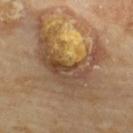Findings:
* workup — no biopsy performed (imaged during a skin exam)
* patient — male, in their 70s
* anatomic site — the upper back
* illumination — cross-polarized
* image-analysis metrics — a lesion color around L≈47 a*≈16 b*≈30 in CIELAB and a normalized border contrast of about 7.5; a border-irregularity index near 10/10, a color-variation rating of about 6/10, and a peripheral color-asymmetry measure near 2; an automated nevus-likeness rating near 0 out of 100 and a lesion-detection confidence of about 0/100
* acquisition — ~15 mm crop, total-body skin-cancer survey
* size — ~7.5 mm (longest diameter)